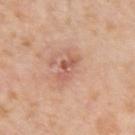Clinical summary: From the left upper arm. A male patient aged 73 to 77. A 15 mm crop from a total-body photograph taken for skin-cancer surveillance. This is a white-light tile. The total-body-photography lesion software estimated a lesion area of about 3.5 mm², a shape eccentricity near 0.9, and a shape-asymmetry score of about 0.45 (0 = symmetric). The software also gave a lesion color around L≈58 a*≈25 b*≈30 in CIELAB, a lesion–skin lightness drop of about 10, and a normalized border contrast of about 6.5. And it measured border irregularity of about 6.5 on a 0–10 scale and a peripheral color-asymmetry measure near 0. The software also gave a nevus-likeness score of about 0/100 and a lesion-detection confidence of about 100/100. Longest diameter approximately 3 mm.